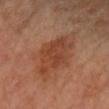* follow-up: imaged on a skin check; not biopsied
* location: the left arm
* subject: female, in their mid-60s
* TBP lesion metrics: a lesion area of about 15 mm² and a symmetry-axis asymmetry near 0.2; border irregularity of about 3 on a 0–10 scale, a within-lesion color-variation index near 3/10, and radial color variation of about 1; an automated nevus-likeness rating near 15 out of 100 and a lesion-detection confidence of about 100/100
* diameter: ~5 mm (longest diameter)
* acquisition: 15 mm crop, total-body photography